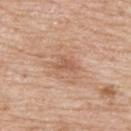biopsy_status: not biopsied; imaged during a skin examination
site: upper back
automated_metrics:
  nevus_likeness_0_100: 0
lighting: white-light
lesion_size:
  long_diameter_mm_approx: 2.5
patient:
  sex: male
  age_approx: 65
image:
  source: total-body photography crop
  field_of_view_mm: 15